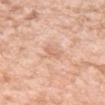– biopsy status — no biopsy performed (imaged during a skin exam)
– imaging modality — total-body-photography crop, ~15 mm field of view
– automated lesion analysis — a lesion area of about 2.5 mm², an outline eccentricity of about 0.9 (0 = round, 1 = elongated), and a symmetry-axis asymmetry near 0.35; a nevus-likeness score of about 0/100 and lesion-presence confidence of about 100/100
– lesion size — about 2.5 mm
– patient — female, aged around 40
– tile lighting — white-light illumination
– body site — the left forearm